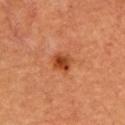Assessment: The lesion was photographed on a routine skin check and not biopsied; there is no pathology result. Acquisition and patient details: A male patient about 65 years old. The recorded lesion diameter is about 2.5 mm. The lesion is located on the chest. A close-up tile cropped from a whole-body skin photograph, about 15 mm across.